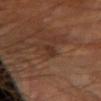Part of a total-body skin-imaging series; this lesion was reviewed on a skin check and was not flagged for biopsy. The lesion is located on the right upper arm. This is a cross-polarized tile. Automated tile analysis of the lesion measured a lesion color around L≈29 a*≈18 b*≈26 in CIELAB and a lesion-to-skin contrast of about 7 (normalized; higher = more distinct). Approximately 2.5 mm at its widest. A 15 mm close-up extracted from a 3D total-body photography capture. A male subject, about 65 years old.